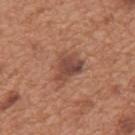follow-up = imaged on a skin check; not biopsied | image = ~15 mm tile from a whole-body skin photo | automated metrics = a lesion color around L≈47 a*≈22 b*≈28 in CIELAB and roughly 10 lightness units darker than nearby skin; a border-irregularity index near 3.5/10, a color-variation rating of about 4.5/10, and peripheral color asymmetry of about 1.5; a nevus-likeness score of about 45/100 | illumination = white-light | subject = male, in their mid- to late 60s | anatomic site = the mid back | size = ~4 mm (longest diameter).